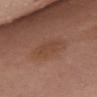Notes:
- biopsy status — total-body-photography surveillance lesion; no biopsy
- image source — total-body-photography crop, ~15 mm field of view
- patient — female, roughly 50 years of age
- lesion size — about 4.5 mm
- TBP lesion metrics — a footprint of about 7 mm² and an outline eccentricity of about 0.9 (0 = round, 1 = elongated); a classifier nevus-likeness of about 0/100 and a lesion-detection confidence of about 100/100
- location — the chest